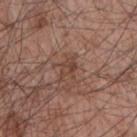Impression: No biopsy was performed on this lesion — it was imaged during a full skin examination and was not determined to be concerning. Acquisition and patient details: The subject is a male roughly 65 years of age. This image is a 15 mm lesion crop taken from a total-body photograph. The lesion is located on the arm.